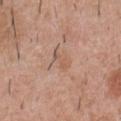Part of a total-body skin-imaging series; this lesion was reviewed on a skin check and was not flagged for biopsy. The tile uses white-light illumination. The total-body-photography lesion software estimated an area of roughly 2.5 mm² and an outline eccentricity of about 0.9 (0 = round, 1 = elongated). And it measured a border-irregularity rating of about 4.5/10, internal color variation of about 0 on a 0–10 scale, and a peripheral color-asymmetry measure near 0. It also reported a classifier nevus-likeness of about 0/100 and a lesion-detection confidence of about 100/100. From the chest. A male subject aged approximately 30. A lesion tile, about 15 mm wide, cut from a 3D total-body photograph.